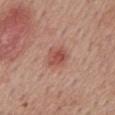Assessment:
Imaged during a routine full-body skin examination; the lesion was not biopsied and no histopathology is available.
Image and clinical context:
On the back. A male subject aged approximately 55. The recorded lesion diameter is about 2.5 mm. The tile uses white-light illumination. A 15 mm crop from a total-body photograph taken for skin-cancer surveillance.